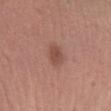acquisition: ~15 mm tile from a whole-body skin photo | site: the left forearm | subject: female, aged 28–32.